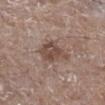Q: Is there a histopathology result?
A: catalogued during a skin exam; not biopsied
Q: What is the imaging modality?
A: 15 mm crop, total-body photography
Q: Lesion location?
A: the right lower leg
Q: Patient demographics?
A: male, aged 63–67